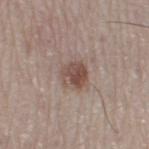A region of skin cropped from a whole-body photographic capture, roughly 15 mm wide.
The subject is a female in their 60s.
The lesion-visualizer software estimated a border-irregularity rating of about 2/10, a within-lesion color-variation index near 5/10, and peripheral color asymmetry of about 2.
The recorded lesion diameter is about 3 mm.
This is a white-light tile.
On the right thigh.